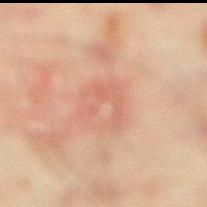The lesion was photographed on a routine skin check and not biopsied; there is no pathology result. A male patient about 45 years old. The total-body-photography lesion software estimated an area of roughly 9.5 mm² and an eccentricity of roughly 0.25. It also reported a lesion–skin lightness drop of about 5. The analysis additionally found a nevus-likeness score of about 0/100 and a lesion-detection confidence of about 100/100. A 15 mm close-up tile from a total-body photography series done for melanoma screening. This is a cross-polarized tile. Longest diameter approximately 4 mm. On the leg.